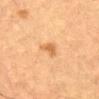{"biopsy_status": "not biopsied; imaged during a skin examination", "lighting": "cross-polarized", "image": {"source": "total-body photography crop", "field_of_view_mm": 15}, "lesion_size": {"long_diameter_mm_approx": 3.0}, "patient": {"sex": "male", "age_approx": "85+"}, "site": "abdomen"}